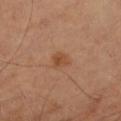lighting: cross-polarized illumination
lesion size: ≈2.5 mm
patient: male, aged 63–67
imaging modality: 15 mm crop, total-body photography
site: the arm